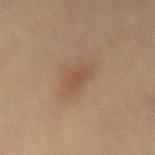Case summary:
– biopsy status · imaged on a skin check; not biopsied
– image · total-body-photography crop, ~15 mm field of view
– patient · female, aged 63 to 67
– site · the lower back